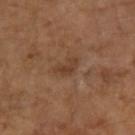Notes:
* follow-up — imaged on a skin check; not biopsied
* subject — female, in their 70s
* tile lighting — cross-polarized illumination
* diameter — ~3 mm (longest diameter)
* TBP lesion metrics — a lesion area of about 4.5 mm², a shape eccentricity near 0.85, and two-axis asymmetry of about 0.35; a lesion color around L≈40 a*≈19 b*≈31 in CIELAB, about 7 CIELAB-L* units darker than the surrounding skin, and a normalized lesion–skin contrast near 6; a border-irregularity index near 3.5/10, a within-lesion color-variation index near 2.5/10, and radial color variation of about 0.5
* anatomic site — the right forearm
* imaging modality — ~15 mm tile from a whole-body skin photo